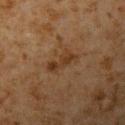Recorded during total-body skin imaging; not selected for excision or biopsy. A male subject aged around 60. A roughly 15 mm field-of-view crop from a total-body skin photograph. Automated tile analysis of the lesion measured a mean CIELAB color near L≈29 a*≈16 b*≈27, roughly 7 lightness units darker than nearby skin, and a lesion-to-skin contrast of about 7.5 (normalized; higher = more distinct). And it measured a border-irregularity index near 5/10, internal color variation of about 1.5 on a 0–10 scale, and peripheral color asymmetry of about 0.5. The lesion is on the left upper arm. About 4 mm across.